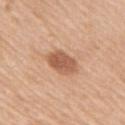Image and clinical context: Measured at roughly 3.5 mm in maximum diameter. A female subject aged 43 to 47. A region of skin cropped from a whole-body photographic capture, roughly 15 mm wide. Imaged with white-light lighting. On the right upper arm. The total-body-photography lesion software estimated a lesion-to-skin contrast of about 8 (normalized; higher = more distinct). It also reported border irregularity of about 1 on a 0–10 scale, a color-variation rating of about 3.5/10, and radial color variation of about 1.